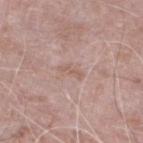Findings:
• site — the left lower leg
• subject — male, roughly 75 years of age
• acquisition — ~15 mm crop, total-body skin-cancer survey